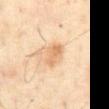Notes:
- follow-up · no biopsy performed (imaged during a skin exam)
- lighting · cross-polarized illumination
- diameter · about 3 mm
- location · the chest
- acquisition · ~15 mm tile from a whole-body skin photo
- patient · male, aged approximately 65